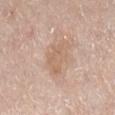Part of a total-body skin-imaging series; this lesion was reviewed on a skin check and was not flagged for biopsy. Cropped from a whole-body photographic skin survey; the tile spans about 15 mm. Approximately 4.5 mm at its widest. The lesion is located on the right lower leg. The patient is a female aged approximately 65. The lesion-visualizer software estimated an outline eccentricity of about 0.85 (0 = round, 1 = elongated) and a shape-asymmetry score of about 0.4 (0 = symmetric). And it measured a color-variation rating of about 2/10 and peripheral color asymmetry of about 0.5. The analysis additionally found a classifier nevus-likeness of about 0/100 and a lesion-detection confidence of about 100/100. This is a white-light tile.